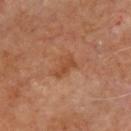Clinical impression:
Captured during whole-body skin photography for melanoma surveillance; the lesion was not biopsied.
Clinical summary:
A male subject, in their mid- to late 60s. Located on the chest. A region of skin cropped from a whole-body photographic capture, roughly 15 mm wide. This is a cross-polarized tile. About 3 mm across.